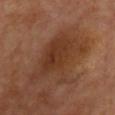The lesion was tiled from a total-body skin photograph and was not biopsied.
The lesion is on the chest.
A 15 mm crop from a total-body photograph taken for skin-cancer surveillance.
A patient approximately 65 years of age.